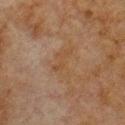Captured during whole-body skin photography for melanoma surveillance; the lesion was not biopsied. Cropped from a whole-body photographic skin survey; the tile spans about 15 mm. The total-body-photography lesion software estimated an area of roughly 5.5 mm², an eccentricity of roughly 0.8, and two-axis asymmetry of about 0.45. And it measured a border-irregularity rating of about 6/10, a within-lesion color-variation index near 2/10, and a peripheral color-asymmetry measure near 0.5. From the front of the torso. A male patient, aged around 80. Approximately 4 mm at its widest. This is a cross-polarized tile.